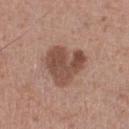Clinical impression: The lesion was tiled from a total-body skin photograph and was not biopsied. Acquisition and patient details: From the left lower leg. A lesion tile, about 15 mm wide, cut from a 3D total-body photograph. The patient is a male aged 53 to 57.